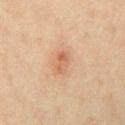The lesion was photographed on a routine skin check and not biopsied; there is no pathology result. The recorded lesion diameter is about 3 mm. The lesion is located on the chest. A male patient, about 40 years old. Cropped from a total-body skin-imaging series; the visible field is about 15 mm.